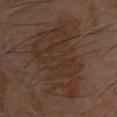{
  "site": "head or neck",
  "lesion_size": {
    "long_diameter_mm_approx": 9.5
  },
  "lighting": "cross-polarized",
  "image": {
    "source": "total-body photography crop",
    "field_of_view_mm": 15
  },
  "patient": {
    "sex": "male",
    "age_approx": 70
  }
}